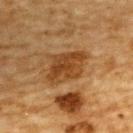Background: From the upper back. A male patient, aged around 85 or older. Approximately 5 mm at its widest. Captured under cross-polarized illumination. A 15 mm close-up tile from a total-body photography series done for melanoma screening.